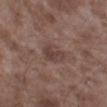No biopsy was performed on this lesion — it was imaged during a full skin examination and was not determined to be concerning. Automated image analysis of the tile measured an area of roughly 6 mm², an eccentricity of roughly 0.75, and a shape-asymmetry score of about 0.25 (0 = symmetric). It also reported a border-irregularity rating of about 3/10, a color-variation rating of about 3/10, and radial color variation of about 1. It also reported a lesion-detection confidence of about 100/100. From the leg. A 15 mm close-up tile from a total-body photography series done for melanoma screening. A male subject aged around 45.